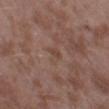The patient is a male aged approximately 45. Located on the left thigh. The total-body-photography lesion software estimated a lesion area of about 3.5 mm², a shape eccentricity near 0.75, and a shape-asymmetry score of about 0.45 (0 = symmetric). It also reported a lesion–skin lightness drop of about 5 and a normalized border contrast of about 4.5. The lesion's longest dimension is about 2.5 mm. A 15 mm close-up tile from a total-body photography series done for melanoma screening. Captured under white-light illumination.